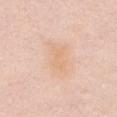Notes:
– follow-up: catalogued during a skin exam; not biopsied
– location: the chest
– illumination: white-light
– subject: female, roughly 50 years of age
– acquisition: ~15 mm tile from a whole-body skin photo